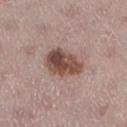| key | value |
|---|---|
| workup | catalogued during a skin exam; not biopsied |
| image | ~15 mm tile from a whole-body skin photo |
| anatomic site | the left lower leg |
| patient | female, aged 43–47 |
| automated metrics | a mean CIELAB color near L≈48 a*≈19 b*≈23, roughly 14 lightness units darker than nearby skin, and a normalized border contrast of about 10.5; a within-lesion color-variation index near 7/10 |